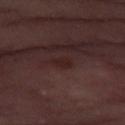notes: no biopsy performed (imaged during a skin exam) | subject: female, in their mid- to late 50s | anatomic site: the right thigh | image source: ~15 mm crop, total-body skin-cancer survey | automated lesion analysis: a footprint of about 3 mm², an outline eccentricity of about 0.9 (0 = round, 1 = elongated), and a symmetry-axis asymmetry near 0.4; a border-irregularity rating of about 4/10, internal color variation of about 0.5 on a 0–10 scale, and peripheral color asymmetry of about 0 | tile lighting: cross-polarized.